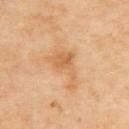Case summary:
- notes: no biopsy performed (imaged during a skin exam)
- patient: male, approximately 65 years of age
- diameter: about 4.5 mm
- location: the upper back
- image: ~15 mm tile from a whole-body skin photo
- illumination: cross-polarized